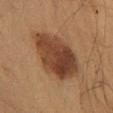This lesion was catalogued during total-body skin photography and was not selected for biopsy. The lesion is on the upper back. A male subject about 60 years old. About 7 mm across. Cropped from a total-body skin-imaging series; the visible field is about 15 mm. Captured under cross-polarized illumination. The lesion-visualizer software estimated an outline eccentricity of about 0.7 (0 = round, 1 = elongated) and a shape-asymmetry score of about 0.2 (0 = symmetric). The software also gave border irregularity of about 2 on a 0–10 scale, internal color variation of about 5.5 on a 0–10 scale, and a peripheral color-asymmetry measure near 2.